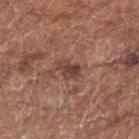biopsy status: no biopsy performed (imaged during a skin exam)
body site: the right forearm
subject: female, aged around 75
image-analysis metrics: a border-irregularity index near 3/10 and a within-lesion color-variation index near 4/10
size: ~3 mm (longest diameter)
image: ~15 mm crop, total-body skin-cancer survey
tile lighting: white-light illumination